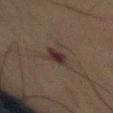follow-up: imaged on a skin check; not biopsied
automated metrics: a lesion–skin lightness drop of about 7 and a normalized lesion–skin contrast near 9.5; a within-lesion color-variation index near 4/10 and radial color variation of about 1; lesion-presence confidence of about 100/100
lesion diameter: about 3.5 mm
body site: the abdomen
acquisition: 15 mm crop, total-body photography
patient: male, in their mid- to late 70s
tile lighting: cross-polarized